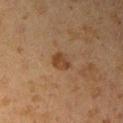The lesion was tiled from a total-body skin photograph and was not biopsied.
The subject is a female aged 18–22.
Located on the right upper arm.
A region of skin cropped from a whole-body photographic capture, roughly 15 mm wide.